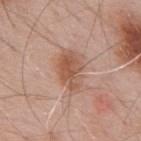Part of a total-body skin-imaging series; this lesion was reviewed on a skin check and was not flagged for biopsy. A male subject aged approximately 55. Longest diameter approximately 5 mm. An algorithmic analysis of the crop reported internal color variation of about 4.5 on a 0–10 scale and radial color variation of about 1.5. The analysis additionally found a nevus-likeness score of about 70/100. Imaged with white-light lighting. Located on the mid back. A close-up tile cropped from a whole-body skin photograph, about 15 mm across.